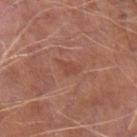Impression:
Part of a total-body skin-imaging series; this lesion was reviewed on a skin check and was not flagged for biopsy.
Context:
The tile uses white-light illumination. A 15 mm close-up tile from a total-body photography series done for melanoma screening. Located on the left upper arm. A male subject aged around 65. The total-body-photography lesion software estimated a footprint of about 3 mm² and a symmetry-axis asymmetry near 0.5.